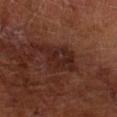Findings:
• follow-up · imaged on a skin check; not biopsied
• acquisition · 15 mm crop, total-body photography
• body site · the left arm
• patient · male, aged 63–67
• automated lesion analysis · a footprint of about 10 mm² and a symmetry-axis asymmetry near 0.4; a lesion color around L≈23 a*≈21 b*≈22 in CIELAB and a lesion-to-skin contrast of about 8 (normalized; higher = more distinct); border irregularity of about 5 on a 0–10 scale and radial color variation of about 1.5